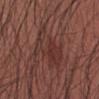workup = total-body-photography surveillance lesion; no biopsy
illumination = white-light
site = the left forearm
automated metrics = two-axis asymmetry of about 0.4; a border-irregularity rating of about 8/10, a within-lesion color-variation index near 2/10, and radial color variation of about 0.5; an automated nevus-likeness rating near 15 out of 100 and lesion-presence confidence of about 95/100
image = 15 mm crop, total-body photography
patient = male, roughly 40 years of age